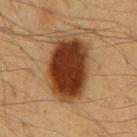workup: total-body-photography surveillance lesion; no biopsy
lighting: cross-polarized
anatomic site: the abdomen
imaging modality: ~15 mm crop, total-body skin-cancer survey
size: ≈8 mm
subject: male, about 60 years old